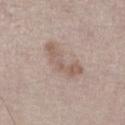Case summary:
– notes · catalogued during a skin exam; not biopsied
– tile lighting · white-light
– patient · male, aged approximately 60
– image-analysis metrics · an outline eccentricity of about 0.9 (0 = round, 1 = elongated) and a symmetry-axis asymmetry near 0.6; border irregularity of about 7.5 on a 0–10 scale and radial color variation of about 0.5; a nevus-likeness score of about 0/100
– acquisition · total-body-photography crop, ~15 mm field of view
– body site · the right lower leg
– size · about 5 mm